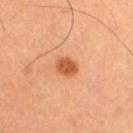This lesion was catalogued during total-body skin photography and was not selected for biopsy. Cropped from a total-body skin-imaging series; the visible field is about 15 mm. Captured under cross-polarized illumination. Automated image analysis of the tile measured a mean CIELAB color near L≈56 a*≈30 b*≈42, roughly 14 lightness units darker than nearby skin, and a lesion-to-skin contrast of about 9 (normalized; higher = more distinct). The analysis additionally found a nevus-likeness score of about 100/100 and a lesion-detection confidence of about 100/100. A male patient roughly 50 years of age. The lesion is on the right thigh.